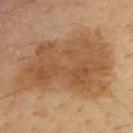Clinical impression:
No biopsy was performed on this lesion — it was imaged during a full skin examination and was not determined to be concerning.
Image and clinical context:
The subject is a male in their mid- to late 40s. The recorded lesion diameter is about 11.5 mm. Captured under cross-polarized illumination. Cropped from a whole-body photographic skin survey; the tile spans about 15 mm. On the upper back.